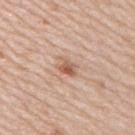Assessment: Part of a total-body skin-imaging series; this lesion was reviewed on a skin check and was not flagged for biopsy. Context: The lesion is located on the upper back. A female patient aged 43 to 47. An algorithmic analysis of the crop reported a shape eccentricity near 0.8. The software also gave border irregularity of about 2.5 on a 0–10 scale, a color-variation rating of about 5.5/10, and radial color variation of about 2. The analysis additionally found a nevus-likeness score of about 85/100. A 15 mm crop from a total-body photograph taken for skin-cancer surveillance.